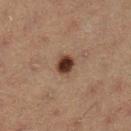* workup — no biopsy performed (imaged during a skin exam)
* tile lighting — cross-polarized
* image — total-body-photography crop, ~15 mm field of view
* diameter — about 2.5 mm
* patient — female, aged 38–42
* location — the leg